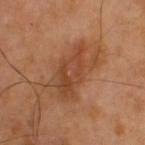Case summary:
• anatomic site: the upper back
• diameter: ≈7 mm
• image-analysis metrics: a lesion area of about 19 mm², an outline eccentricity of about 0.85 (0 = round, 1 = elongated), and two-axis asymmetry of about 0.3; border irregularity of about 6 on a 0–10 scale and a peripheral color-asymmetry measure near 1.5
• subject: male, aged around 40
• imaging modality: 15 mm crop, total-body photography
• lighting: cross-polarized